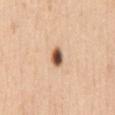Impression:
The lesion was tiled from a total-body skin photograph and was not biopsied.
Image and clinical context:
This image is a 15 mm lesion crop taken from a total-body photograph. The lesion is on the front of the torso. This is a white-light tile. Longest diameter approximately 2.5 mm. The subject is a female aged 28–32.